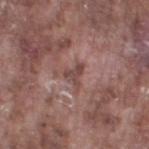<record>
  <biopsy_status>not biopsied; imaged during a skin examination</biopsy_status>
  <image>
    <source>total-body photography crop</source>
    <field_of_view_mm>15</field_of_view_mm>
  </image>
  <site>left thigh</site>
  <patient>
    <sex>male</sex>
    <age_approx>75</age_approx>
  </patient>
</record>